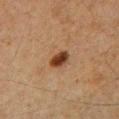The lesion was biopsied, and histopathology showed a dysplastic (Clark) nevus — a benign skin lesion.